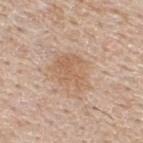A 15 mm close-up extracted from a 3D total-body photography capture.
Measured at roughly 4.5 mm in maximum diameter.
Captured under white-light illumination.
Automated tile analysis of the lesion measured an area of roughly 12 mm², a shape eccentricity near 0.65, and a shape-asymmetry score of about 0.3 (0 = symmetric). The analysis additionally found an average lesion color of about L≈61 a*≈18 b*≈32 (CIELAB), a lesion–skin lightness drop of about 7, and a lesion-to-skin contrast of about 5.5 (normalized; higher = more distinct). The software also gave an automated nevus-likeness rating near 0 out of 100 and a detector confidence of about 100 out of 100 that the crop contains a lesion.
A male subject, approximately 55 years of age.
The lesion is located on the upper back.